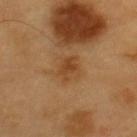No biopsy was performed on this lesion — it was imaged during a full skin examination and was not determined to be concerning. A male patient aged approximately 60. The lesion is located on the upper back. A roughly 15 mm field-of-view crop from a total-body skin photograph. Captured under cross-polarized illumination. The recorded lesion diameter is about 3 mm.